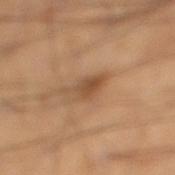{
  "biopsy_status": "not biopsied; imaged during a skin examination",
  "image": {
    "source": "total-body photography crop",
    "field_of_view_mm": 15
  },
  "patient": {
    "sex": "male",
    "age_approx": 40
  },
  "site": "right lower leg"
}